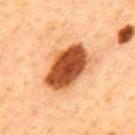{"biopsy_status": "not biopsied; imaged during a skin examination", "site": "mid back", "lesion_size": {"long_diameter_mm_approx": 6.0}, "lighting": "cross-polarized", "image": {"source": "total-body photography crop", "field_of_view_mm": 15}, "automated_metrics": {"area_mm2_approx": 20.0, "shape_asymmetry": 0.15, "nevus_likeness_0_100": 100, "lesion_detection_confidence_0_100": 100}, "patient": {"sex": "male", "age_approx": 50}}